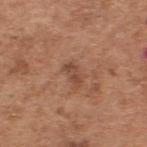| key | value |
|---|---|
| notes | imaged on a skin check; not biopsied |
| body site | the back |
| automated lesion analysis | a border-irregularity rating of about 3.5/10, a color-variation rating of about 3/10, and radial color variation of about 1; an automated nevus-likeness rating near 0 out of 100 and a lesion-detection confidence of about 100/100 |
| patient | male, roughly 65 years of age |
| image source | ~15 mm crop, total-body skin-cancer survey |
| illumination | white-light illumination |